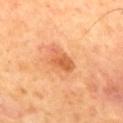workup: no biopsy performed (imaged during a skin exam) | acquisition: 15 mm crop, total-body photography | patient: male, aged 63–67 | lesion diameter: ~3.5 mm (longest diameter) | location: the mid back.